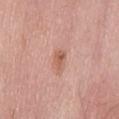{"biopsy_status": "not biopsied; imaged during a skin examination", "lesion_size": {"long_diameter_mm_approx": 2.5}, "site": "mid back", "automated_metrics": {"cielab_L": 58, "cielab_a": 24, "cielab_b": 29, "vs_skin_darker_L": 10.0, "vs_skin_contrast_norm": 7.0}, "patient": {"sex": "male", "age_approx": 70}, "image": {"source": "total-body photography crop", "field_of_view_mm": 15}}